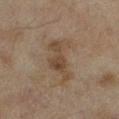Findings:
* notes — no biopsy performed (imaged during a skin exam)
* image source — total-body-photography crop, ~15 mm field of view
* patient — female, aged around 60
* size — ≈5.5 mm
* lighting — cross-polarized
* anatomic site — the leg